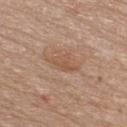Clinical impression: This lesion was catalogued during total-body skin photography and was not selected for biopsy. Background: Imaged with white-light lighting. Located on the chest. Cropped from a whole-body photographic skin survey; the tile spans about 15 mm. Automated tile analysis of the lesion measured an area of roughly 4.5 mm², an outline eccentricity of about 0.85 (0 = round, 1 = elongated), and a symmetry-axis asymmetry near 0.4. It also reported a mean CIELAB color near L≈53 a*≈20 b*≈31, about 7 CIELAB-L* units darker than the surrounding skin, and a normalized lesion–skin contrast near 6. It also reported a nevus-likeness score of about 0/100 and a lesion-detection confidence of about 100/100. A female subject approximately 35 years of age. Measured at roughly 3.5 mm in maximum diameter.